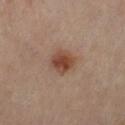No biopsy was performed on this lesion — it was imaged during a full skin examination and was not determined to be concerning.
Longest diameter approximately 3 mm.
This image is a 15 mm lesion crop taken from a total-body photograph.
A female subject roughly 50 years of age.
Captured under cross-polarized illumination.
The lesion is on the left thigh.